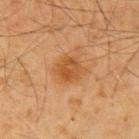{
  "lighting": "cross-polarized",
  "site": "right upper arm",
  "patient": {
    "sex": "male",
    "age_approx": 70
  },
  "image": {
    "source": "total-body photography crop",
    "field_of_view_mm": 15
  }
}